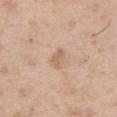Q: Was a biopsy performed?
A: total-body-photography surveillance lesion; no biopsy
Q: Who is the patient?
A: male, aged around 50
Q: Where on the body is the lesion?
A: the right thigh
Q: What is the imaging modality?
A: ~15 mm crop, total-body skin-cancer survey